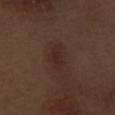Q: Is there a histopathology result?
A: no biopsy performed (imaged during a skin exam)
Q: Lesion location?
A: the left thigh
Q: What did automated image analysis measure?
A: a lesion area of about 5 mm² and a symmetry-axis asymmetry near 0.4; a nevus-likeness score of about 40/100
Q: What kind of image is this?
A: ~15 mm crop, total-body skin-cancer survey
Q: What lighting was used for the tile?
A: white-light illumination
Q: Who is the patient?
A: male, aged around 70
Q: Lesion size?
A: ~3.5 mm (longest diameter)